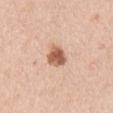<case>
  <biopsy_status>not biopsied; imaged during a skin examination</biopsy_status>
  <patient>
    <sex>male</sex>
    <age_approx>50</age_approx>
  </patient>
  <lesion_size>
    <long_diameter_mm_approx>3.0</long_diameter_mm_approx>
  </lesion_size>
  <image>
    <source>total-body photography crop</source>
    <field_of_view_mm>15</field_of_view_mm>
  </image>
  <site>right upper arm</site>
</case>